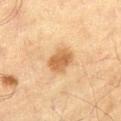Assessment:
Captured during whole-body skin photography for melanoma surveillance; the lesion was not biopsied.
Image and clinical context:
The lesion is located on the abdomen. Automated tile analysis of the lesion measured a lesion color around L≈51 a*≈18 b*≈35 in CIELAB, about 11 CIELAB-L* units darker than the surrounding skin, and a normalized border contrast of about 8. And it measured a classifier nevus-likeness of about 90/100 and lesion-presence confidence of about 100/100. The lesion's longest dimension is about 3.5 mm. This image is a 15 mm lesion crop taken from a total-body photograph. A male patient roughly 70 years of age. The tile uses cross-polarized illumination.